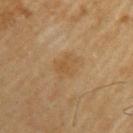Q: Is there a histopathology result?
A: total-body-photography surveillance lesion; no biopsy
Q: Automated lesion metrics?
A: an average lesion color of about L≈49 a*≈15 b*≈36 (CIELAB) and about 6 CIELAB-L* units darker than the surrounding skin
Q: Lesion location?
A: the left upper arm
Q: What kind of image is this?
A: 15 mm crop, total-body photography
Q: Patient demographics?
A: male, aged approximately 70
Q: How was the tile lit?
A: cross-polarized illumination